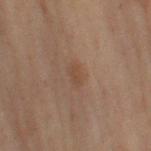Measured at roughly 2.5 mm in maximum diameter.
The lesion-visualizer software estimated roughly 4 lightness units darker than nearby skin and a normalized lesion–skin contrast near 4.5. And it measured a lesion-detection confidence of about 100/100.
Imaged with cross-polarized lighting.
From the left upper arm.
The patient is a male about 70 years old.
A 15 mm close-up extracted from a 3D total-body photography capture.